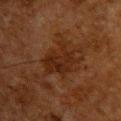workup: catalogued during a skin exam; not biopsied
TBP lesion metrics: a footprint of about 14 mm² and an outline eccentricity of about 0.75 (0 = round, 1 = elongated); a nevus-likeness score of about 0/100 and a detector confidence of about 100 out of 100 that the crop contains a lesion
patient: male, about 60 years old
site: the chest
imaging modality: ~15 mm crop, total-body skin-cancer survey